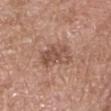Q: Was this lesion biopsied?
A: no biopsy performed (imaged during a skin exam)
Q: How was this image acquired?
A: 15 mm crop, total-body photography
Q: How large is the lesion?
A: ≈4.5 mm
Q: How was the tile lit?
A: white-light
Q: Automated lesion metrics?
A: a lesion area of about 11 mm² and a shape-asymmetry score of about 0.3 (0 = symmetric); about 9 CIELAB-L* units darker than the surrounding skin and a normalized lesion–skin contrast near 6.5; a classifier nevus-likeness of about 0/100 and a detector confidence of about 100 out of 100 that the crop contains a lesion
Q: What is the anatomic site?
A: the head or neck
Q: What are the patient's age and sex?
A: male, roughly 65 years of age